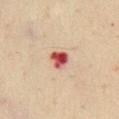Captured under cross-polarized illumination.
A lesion tile, about 15 mm wide, cut from a 3D total-body photograph.
The lesion is located on the chest.
A male patient in their 50s.
The recorded lesion diameter is about 2.5 mm.
Automated image analysis of the tile measured a lesion color around L≈48 a*≈31 b*≈26 in CIELAB and a lesion-to-skin contrast of about 12.5 (normalized; higher = more distinct).